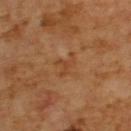biopsy_status: not biopsied; imaged during a skin examination
automated_metrics:
  eccentricity: 0.7
  shape_asymmetry: 0.45
  cielab_L: 42
  cielab_a: 22
  cielab_b: 35
  vs_skin_darker_L: 6.0
  vs_skin_contrast_norm: 5.0
  border_irregularity_0_10: 5.5
  color_variation_0_10: 1.0
  peripheral_color_asymmetry: 0.5
site: upper back
lesion_size:
  long_diameter_mm_approx: 3.0
lighting: cross-polarized
image:
  source: total-body photography crop
  field_of_view_mm: 15
patient:
  sex: male
  age_approx: 65